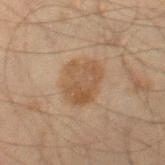Context:
Imaged with cross-polarized lighting. A region of skin cropped from a whole-body photographic capture, roughly 15 mm wide. The patient is a male roughly 60 years of age. The lesion is on the right thigh.